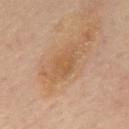Q: How was this image acquired?
A: total-body-photography crop, ~15 mm field of view
Q: Lesion location?
A: the mid back
Q: Who is the patient?
A: male, aged 63–67